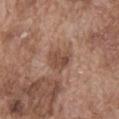No biopsy was performed on this lesion — it was imaged during a full skin examination and was not determined to be concerning.
Imaged with white-light lighting.
On the abdomen.
About 3 mm across.
A male patient about 75 years old.
An algorithmic analysis of the crop reported an area of roughly 6 mm² and a shape eccentricity near 0.5. It also reported a normalized lesion–skin contrast near 7. The analysis additionally found a classifier nevus-likeness of about 0/100 and a lesion-detection confidence of about 100/100.
A roughly 15 mm field-of-view crop from a total-body skin photograph.